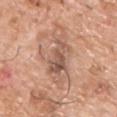Clinical summary:
A region of skin cropped from a whole-body photographic capture, roughly 15 mm wide. Imaged with white-light lighting. Longest diameter approximately 4 mm. A male subject, in their mid- to late 70s. The lesion is located on the upper back.